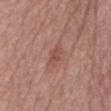  biopsy_status: not biopsied; imaged during a skin examination
  lighting: white-light
  site: mid back
  lesion_size:
    long_diameter_mm_approx: 3.0
  image:
    source: total-body photography crop
    field_of_view_mm: 15
  patient:
    sex: female
    age_approx: 75